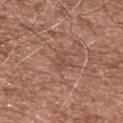Impression: The lesion was photographed on a routine skin check and not biopsied; there is no pathology result.